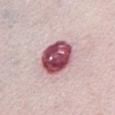Part of a total-body skin-imaging series; this lesion was reviewed on a skin check and was not flagged for biopsy.
A 15 mm close-up tile from a total-body photography series done for melanoma screening.
The tile uses white-light illumination.
The lesion is on the chest.
The patient is a female about 65 years old.